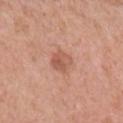This lesion was catalogued during total-body skin photography and was not selected for biopsy. The lesion is located on the arm. Imaged with white-light lighting. A female subject, roughly 60 years of age. About 2.5 mm across. A lesion tile, about 15 mm wide, cut from a 3D total-body photograph.